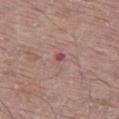Clinical impression: Part of a total-body skin-imaging series; this lesion was reviewed on a skin check and was not flagged for biopsy. Context: Measured at roughly 3 mm in maximum diameter. A close-up tile cropped from a whole-body skin photograph, about 15 mm across. A male patient, aged approximately 65. The tile uses white-light illumination. The lesion is on the left thigh.